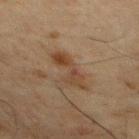No biopsy was performed on this lesion — it was imaged during a full skin examination and was not determined to be concerning.